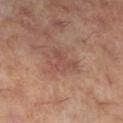The lesion is on the right lower leg. Longest diameter approximately 3.5 mm. A roughly 15 mm field-of-view crop from a total-body skin photograph. This is a cross-polarized tile. A female patient, aged 58–62.